Cropped from a whole-body photographic skin survey; the tile spans about 15 mm. Captured under white-light illumination. Measured at roughly 8.5 mm in maximum diameter. The subject is a male in their 70s. On the mid back. An algorithmic analysis of the crop reported a lesion area of about 29 mm², a shape eccentricity near 0.85, and a shape-asymmetry score of about 0.15 (0 = symmetric). The software also gave a mean CIELAB color near L≈43 a*≈14 b*≈17, about 16 CIELAB-L* units darker than the surrounding skin, and a normalized lesion–skin contrast near 13. It also reported border irregularity of about 2.5 on a 0–10 scale, a color-variation rating of about 5.5/10, and radial color variation of about 2.
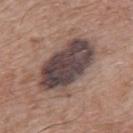Conclusion:
The biopsy diagnosis was a benign skin lesion: dysplastic (Clark) nevus.